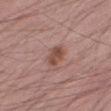Imaged during a routine full-body skin examination; the lesion was not biopsied and no histopathology is available. Approximately 3 mm at its widest. The patient is a male about 55 years old. The lesion is located on the right thigh. A lesion tile, about 15 mm wide, cut from a 3D total-body photograph. Automated tile analysis of the lesion measured roughly 10 lightness units darker than nearby skin. The analysis additionally found a nevus-likeness score of about 65/100 and lesion-presence confidence of about 100/100. The tile uses white-light illumination.